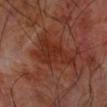A 15 mm close-up tile from a total-body photography series done for melanoma screening.
The lesion is located on the left forearm.
The tile uses cross-polarized illumination.
The recorded lesion diameter is about 7 mm.
The subject is a male roughly 70 years of age.